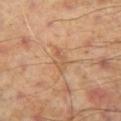| feature | finding |
|---|---|
| biopsy status | no biopsy performed (imaged during a skin exam) |
| automated metrics | a footprint of about 3 mm² and two-axis asymmetry of about 0.4; border irregularity of about 5.5 on a 0–10 scale, a color-variation rating of about 0/10, and a peripheral color-asymmetry measure near 0; a nevus-likeness score of about 0/100 and a detector confidence of about 95 out of 100 that the crop contains a lesion |
| lesion size | ~3 mm (longest diameter) |
| body site | the left thigh |
| image source | 15 mm crop, total-body photography |
| illumination | cross-polarized illumination |
| subject | male, in their mid- to late 50s |